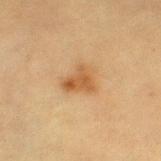workup: no biopsy performed (imaged during a skin exam) | lighting: cross-polarized | site: the leg | image: ~15 mm tile from a whole-body skin photo | subject: female, aged approximately 55 | image-analysis metrics: an area of roughly 7 mm² and a shape-asymmetry score of about 0.45 (0 = symmetric); a lesion color around L≈48 a*≈18 b*≈36 in CIELAB, roughly 9 lightness units darker than nearby skin, and a normalized border contrast of about 7.5; an automated nevus-likeness rating near 80 out of 100 and a lesion-detection confidence of about 100/100 | lesion diameter: ~3.5 mm (longest diameter).